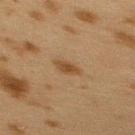workup — catalogued during a skin exam; not biopsied | tile lighting — cross-polarized illumination | image — ~15 mm tile from a whole-body skin photo | patient — female, aged approximately 40 | diameter — about 2.5 mm | location — the upper back.